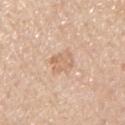Image and clinical context: A male subject in their mid- to late 50s. The lesion is on the chest. This image is a 15 mm lesion crop taken from a total-body photograph.